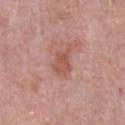Captured during whole-body skin photography for melanoma surveillance; the lesion was not biopsied. Automated tile analysis of the lesion measured an average lesion color of about L≈54 a*≈25 b*≈27 (CIELAB) and a normalized border contrast of about 6.5. It also reported a border-irregularity index near 4/10 and a color-variation rating of about 2.5/10. And it measured a nevus-likeness score of about 0/100. Located on the chest. Approximately 3.5 mm at its widest. This is a white-light tile. A male patient, aged 48–52. Cropped from a whole-body photographic skin survey; the tile spans about 15 mm.